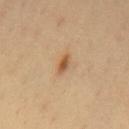| field | value |
|---|---|
| notes | imaged on a skin check; not biopsied |
| subject | male, roughly 40 years of age |
| image | total-body-photography crop, ~15 mm field of view |
| TBP lesion metrics | a border-irregularity rating of about 3.5/10, a within-lesion color-variation index near 0/10, and radial color variation of about 0; an automated nevus-likeness rating near 85 out of 100 and a detector confidence of about 100 out of 100 that the crop contains a lesion |
| tile lighting | cross-polarized illumination |
| body site | the mid back |